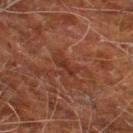Clinical impression:
The lesion was photographed on a routine skin check and not biopsied; there is no pathology result.
Image and clinical context:
An algorithmic analysis of the crop reported border irregularity of about 4.5 on a 0–10 scale, a color-variation rating of about 0/10, and radial color variation of about 0. The analysis additionally found a classifier nevus-likeness of about 0/100. The tile uses cross-polarized illumination. The recorded lesion diameter is about 2.5 mm. A region of skin cropped from a whole-body photographic capture, roughly 15 mm wide. The lesion is on the right leg. A male patient, aged 58–62.